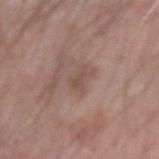An algorithmic analysis of the crop reported a lesion area of about 2.5 mm², an outline eccentricity of about 0.85 (0 = round, 1 = elongated), and a symmetry-axis asymmetry near 0.4. The software also gave border irregularity of about 4.5 on a 0–10 scale and internal color variation of about 0 on a 0–10 scale. A region of skin cropped from a whole-body photographic capture, roughly 15 mm wide. Longest diameter approximately 2.5 mm. This is a white-light tile. A male subject aged 63–67. On the left forearm.